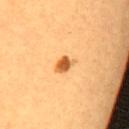Imaged during a routine full-body skin examination; the lesion was not biopsied and no histopathology is available.
The recorded lesion diameter is about 2.5 mm.
From the upper back.
This image is a 15 mm lesion crop taken from a total-body photograph.
The patient is a female aged 28 to 32.
Automated tile analysis of the lesion measured an area of roughly 3.5 mm², a shape eccentricity near 0.75, and a shape-asymmetry score of about 0.3 (0 = symmetric). And it measured a lesion color around L≈57 a*≈26 b*≈46 in CIELAB, about 15 CIELAB-L* units darker than the surrounding skin, and a lesion-to-skin contrast of about 9.5 (normalized; higher = more distinct). And it measured a border-irregularity index near 2.5/10 and a peripheral color-asymmetry measure near 1.